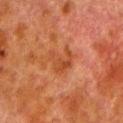| field | value |
|---|---|
| workup | total-body-photography surveillance lesion; no biopsy |
| body site | the leg |
| lesion size | ~3.5 mm (longest diameter) |
| imaging modality | ~15 mm crop, total-body skin-cancer survey |
| patient | male, aged around 80 |